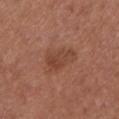Captured during whole-body skin photography for melanoma surveillance; the lesion was not biopsied.
Approximately 4.5 mm at its widest.
A 15 mm crop from a total-body photograph taken for skin-cancer surveillance.
From the left lower leg.
The tile uses white-light illumination.
The patient is a female aged 53 to 57.